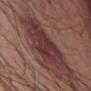Impression:
Captured during whole-body skin photography for melanoma surveillance; the lesion was not biopsied.
Clinical summary:
From the upper back. A 15 mm close-up tile from a total-body photography series done for melanoma screening. The tile uses white-light illumination. A male patient, in their mid- to late 40s. About 8 mm across.